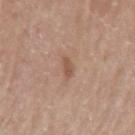notes: imaged on a skin check; not biopsied
subject: male, aged 68–72
size: about 2.5 mm
site: the arm
image source: ~15 mm crop, total-body skin-cancer survey